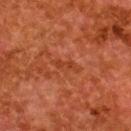notes: no biopsy performed (imaged during a skin exam)
site: the upper back
acquisition: total-body-photography crop, ~15 mm field of view
image-analysis metrics: a footprint of about 2.5 mm², an eccentricity of roughly 0.95, and a shape-asymmetry score of about 0.55 (0 = symmetric); a border-irregularity index near 6.5/10 and radial color variation of about 0; a nevus-likeness score of about 0/100 and a detector confidence of about 100 out of 100 that the crop contains a lesion
tile lighting: cross-polarized
patient: female, aged 48 to 52